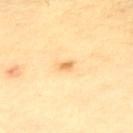• notes · total-body-photography surveillance lesion; no biopsy
• lighting · cross-polarized
• patient · male, aged approximately 70
• location · the upper back
• diameter · ≈2 mm
• automated lesion analysis · a footprint of about 2 mm² and a shape eccentricity near 0.8; a mean CIELAB color near L≈73 a*≈20 b*≈47, a lesion–skin lightness drop of about 11, and a normalized border contrast of about 7; a detector confidence of about 100 out of 100 that the crop contains a lesion
• image · ~15 mm crop, total-body skin-cancer survey